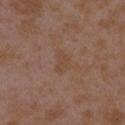{"biopsy_status": "not biopsied; imaged during a skin examination", "patient": {"sex": "female", "age_approx": 35}, "lighting": "white-light", "image": {"source": "total-body photography crop", "field_of_view_mm": 15}, "lesion_size": {"long_diameter_mm_approx": 3.0}, "automated_metrics": {"shape_asymmetry": 0.35, "nevus_likeness_0_100": 0, "lesion_detection_confidence_0_100": 100}, "site": "arm"}